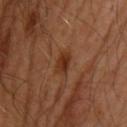Case summary:
• notes — imaged on a skin check; not biopsied
• patient — male, about 40 years old
• illumination — cross-polarized illumination
• image source — ~15 mm tile from a whole-body skin photo
• automated metrics — a border-irregularity index near 2.5/10, a color-variation rating of about 3/10, and radial color variation of about 1; an automated nevus-likeness rating near 90 out of 100
• size — ≈3 mm
• anatomic site — the upper back